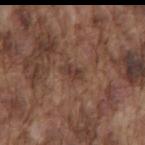Impression:
Part of a total-body skin-imaging series; this lesion was reviewed on a skin check and was not flagged for biopsy.
Clinical summary:
The patient is a male roughly 75 years of age. Automated image analysis of the tile measured border irregularity of about 2.5 on a 0–10 scale. The software also gave a lesion-detection confidence of about 95/100. This image is a 15 mm lesion crop taken from a total-body photograph. From the mid back.